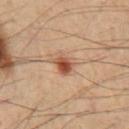Assessment:
Captured during whole-body skin photography for melanoma surveillance; the lesion was not biopsied.
Acquisition and patient details:
A male subject, aged around 60. Longest diameter approximately 3 mm. From the chest. A 15 mm close-up tile from a total-body photography series done for melanoma screening. The tile uses cross-polarized illumination.